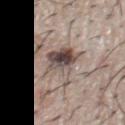Assessment: Recorded during total-body skin imaging; not selected for excision or biopsy. Image and clinical context: A male patient approximately 65 years of age. The lesion is on the chest. Cropped from a whole-body photographic skin survey; the tile spans about 15 mm.